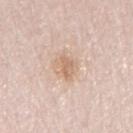<tbp_lesion>
<biopsy_status>not biopsied; imaged during a skin examination</biopsy_status>
<patient>
  <sex>male</sex>
  <age_approx>65</age_approx>
</patient>
<image>
  <source>total-body photography crop</source>
  <field_of_view_mm>15</field_of_view_mm>
</image>
<site>right upper arm</site>
<lighting>white-light</lighting>
<lesion_size>
  <long_diameter_mm_approx>3.5</long_diameter_mm_approx>
</lesion_size>
</tbp_lesion>